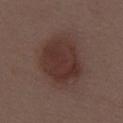Imaged during a routine full-body skin examination; the lesion was not biopsied and no histopathology is available.
The total-body-photography lesion software estimated a border-irregularity index near 2/10, a color-variation rating of about 3/10, and radial color variation of about 1.
This is a white-light tile.
The lesion is on the abdomen.
This image is a 15 mm lesion crop taken from a total-body photograph.
The patient is a female aged around 50.
The lesion's longest dimension is about 6 mm.